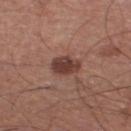workup=no biopsy performed (imaged during a skin exam); diameter=~3.5 mm (longest diameter); patient=male, aged around 65; lighting=white-light; acquisition=15 mm crop, total-body photography; site=the right thigh.